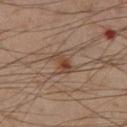The lesion was photographed on a routine skin check and not biopsied; there is no pathology result. The subject is a male about 55 years old. A lesion tile, about 15 mm wide, cut from a 3D total-body photograph. Captured under cross-polarized illumination. Located on the left thigh. An algorithmic analysis of the crop reported an area of roughly 4.5 mm², an outline eccentricity of about 0.85 (0 = round, 1 = elongated), and a symmetry-axis asymmetry near 0.3. And it measured a lesion–skin lightness drop of about 9 and a lesion-to-skin contrast of about 7.5 (normalized; higher = more distinct). The software also gave a border-irregularity rating of about 3/10, a within-lesion color-variation index near 6.5/10, and peripheral color asymmetry of about 2.5. And it measured an automated nevus-likeness rating near 60 out of 100 and lesion-presence confidence of about 100/100. Measured at roughly 3 mm in maximum diameter.